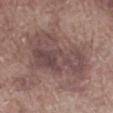Case summary:
• follow-up: imaged on a skin check; not biopsied
• subject: male, approximately 70 years of age
• size: about 8 mm
• anatomic site: the left lower leg
• imaging modality: ~15 mm crop, total-body skin-cancer survey
• lighting: white-light illumination